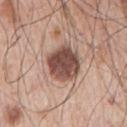Captured during whole-body skin photography for melanoma surveillance; the lesion was not biopsied. On the arm. A male patient about 65 years old. The tile uses white-light illumination. A close-up tile cropped from a whole-body skin photograph, about 15 mm across. The lesion-visualizer software estimated an eccentricity of roughly 0.6 and a symmetry-axis asymmetry near 0.15. The analysis additionally found a lesion–skin lightness drop of about 18. It also reported a classifier nevus-likeness of about 30/100 and a lesion-detection confidence of about 100/100. Longest diameter approximately 4.5 mm.